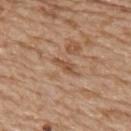biopsy_status: not biopsied; imaged during a skin examination
patient:
  sex: female
  age_approx: 70
site: upper back
lesion_size:
  long_diameter_mm_approx: 3.0
image:
  source: total-body photography crop
  field_of_view_mm: 15
lighting: white-light